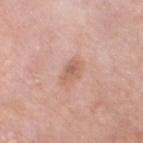workup: catalogued during a skin exam; not biopsied | location: the left lower leg | subject: male, aged around 80 | image: 15 mm crop, total-body photography | illumination: white-light illumination | lesion size: about 3 mm.